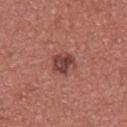{
  "biopsy_status": "not biopsied; imaged during a skin examination",
  "lighting": "white-light",
  "lesion_size": {
    "long_diameter_mm_approx": 3.0
  },
  "patient": {
    "sex": "male",
    "age_approx": 40
  },
  "image": {
    "source": "total-body photography crop",
    "field_of_view_mm": 15
  },
  "site": "upper back",
  "automated_metrics": {
    "cielab_L": 43,
    "cielab_a": 25,
    "cielab_b": 24,
    "vs_skin_darker_L": 11.0,
    "vs_skin_contrast_norm": 8.5,
    "peripheral_color_asymmetry": 1.5
  }
}